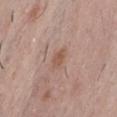Notes:
– biopsy status: total-body-photography surveillance lesion; no biopsy